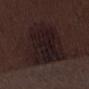{
  "biopsy_status": "not biopsied; imaged during a skin examination",
  "lesion_size": {
    "long_diameter_mm_approx": 7.5
  },
  "site": "left thigh",
  "patient": {
    "sex": "male",
    "age_approx": 70
  },
  "lighting": "white-light",
  "image": {
    "source": "total-body photography crop",
    "field_of_view_mm": 15
  }
}